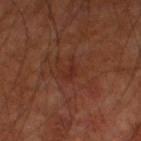Captured during whole-body skin photography for melanoma surveillance; the lesion was not biopsied. Cropped from a whole-body photographic skin survey; the tile spans about 15 mm. Approximately 2.5 mm at its widest. A male patient aged 58–62. The lesion is on the left thigh.